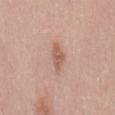Measured at roughly 4 mm in maximum diameter. A female subject, about 30 years old. From the mid back. This is a white-light tile. A 15 mm close-up extracted from a 3D total-body photography capture.